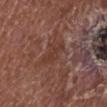No biopsy was performed on this lesion — it was imaged during a full skin examination and was not determined to be concerning. A lesion tile, about 15 mm wide, cut from a 3D total-body photograph. The lesion is located on the left lower leg. Automated image analysis of the tile measured a nevus-likeness score of about 0/100 and lesion-presence confidence of about 85/100. A male patient, in their mid-70s. This is a white-light tile.